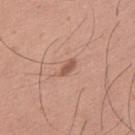Clinical impression:
Part of a total-body skin-imaging series; this lesion was reviewed on a skin check and was not flagged for biopsy.
Clinical summary:
Imaged with white-light lighting. Approximately 2.5 mm at its widest. A male subject, aged 33 to 37. A lesion tile, about 15 mm wide, cut from a 3D total-body photograph. On the back.